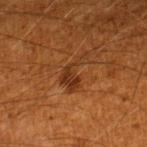Clinical impression:
No biopsy was performed on this lesion — it was imaged during a full skin examination and was not determined to be concerning.
Acquisition and patient details:
Cropped from a total-body skin-imaging series; the visible field is about 15 mm. Longest diameter approximately 4.5 mm. The subject is a male aged approximately 60. The lesion is on the leg. The lesion-visualizer software estimated a footprint of about 6.5 mm². The software also gave border irregularity of about 8 on a 0–10 scale and a color-variation rating of about 3/10.